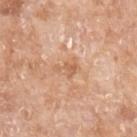| key | value |
|---|---|
| workup | catalogued during a skin exam; not biopsied |
| diameter | about 3 mm |
| lighting | white-light |
| subject | female, aged approximately 75 |
| imaging modality | ~15 mm crop, total-body skin-cancer survey |
| anatomic site | the left forearm |